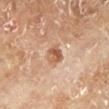The lesion was photographed on a routine skin check and not biopsied; there is no pathology result. This is a cross-polarized tile. This image is a 15 mm lesion crop taken from a total-body photograph. The lesion-visualizer software estimated a border-irregularity index near 2/10 and radial color variation of about 2.5. The recorded lesion diameter is about 2.5 mm. A male patient, aged around 85 or older. From the leg.